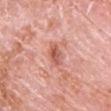Impression: No biopsy was performed on this lesion — it was imaged during a full skin examination and was not determined to be concerning. Clinical summary: The recorded lesion diameter is about 4 mm. An algorithmic analysis of the crop reported a lesion color around L≈60 a*≈28 b*≈30 in CIELAB. The analysis additionally found a border-irregularity rating of about 2.5/10, a within-lesion color-variation index near 6/10, and peripheral color asymmetry of about 2. Imaged with white-light lighting. Located on the front of the torso. A male patient, aged around 80. A close-up tile cropped from a whole-body skin photograph, about 15 mm across.